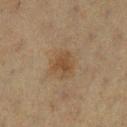Approximately 2.5 mm at its widest.
The subject is a female roughly 55 years of age.
Cropped from a whole-body photographic skin survey; the tile spans about 15 mm.
Imaged with cross-polarized lighting.
The lesion is located on the left lower leg.